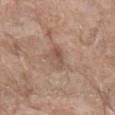follow-up — total-body-photography surveillance lesion; no biopsy | image-analysis metrics — an outline eccentricity of about 0.9 (0 = round, 1 = elongated) and a symmetry-axis asymmetry near 0.3; lesion-presence confidence of about 100/100 | diameter — about 3.5 mm | imaging modality — ~15 mm tile from a whole-body skin photo | lighting — white-light | patient — female, about 75 years old | anatomic site — the left forearm.